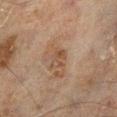Imaged during a routine full-body skin examination; the lesion was not biopsied and no histopathology is available. A roughly 15 mm field-of-view crop from a total-body skin photograph. About 2.5 mm across. The total-body-photography lesion software estimated a border-irregularity index near 3/10, internal color variation of about 3.5 on a 0–10 scale, and peripheral color asymmetry of about 1.5. The analysis additionally found lesion-presence confidence of about 100/100. A male patient, aged 58 to 62. Located on the left lower leg.